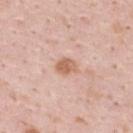Image and clinical context:
A male patient, about 35 years old. Approximately 2.5 mm at its widest. Cropped from a whole-body photographic skin survey; the tile spans about 15 mm. Located on the upper back.